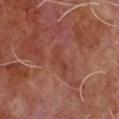  biopsy_status: not biopsied; imaged during a skin examination
  lighting: cross-polarized
  image:
    source: total-body photography crop
    field_of_view_mm: 15
  site: chest
  lesion_size:
    long_diameter_mm_approx: 4.0
  patient:
    sex: male
    age_approx: 65